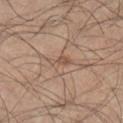Clinical impression: This lesion was catalogued during total-body skin photography and was not selected for biopsy. Clinical summary: The lesion is on the right lower leg. A male patient in their mid- to late 40s. The lesion's longest dimension is about 3 mm. Imaged with white-light lighting. A 15 mm close-up tile from a total-body photography series done for melanoma screening. Automated tile analysis of the lesion measured a lesion color around L≈53 a*≈18 b*≈29 in CIELAB and a normalized lesion–skin contrast near 6. And it measured a peripheral color-asymmetry measure near 0. It also reported a lesion-detection confidence of about 70/100.